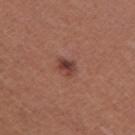Assessment:
Part of a total-body skin-imaging series; this lesion was reviewed on a skin check and was not flagged for biopsy.
Context:
The lesion is on the left thigh. The total-body-photography lesion software estimated a lesion color around L≈41 a*≈24 b*≈25 in CIELAB and a normalized lesion–skin contrast near 8.5. The software also gave border irregularity of about 2.5 on a 0–10 scale, a color-variation rating of about 4.5/10, and radial color variation of about 1.5. The software also gave a detector confidence of about 100 out of 100 that the crop contains a lesion. Captured under white-light illumination. A 15 mm close-up tile from a total-body photography series done for melanoma screening. The recorded lesion diameter is about 2.5 mm. The patient is a female aged 43–47.